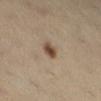| feature | finding |
|---|---|
| workup | catalogued during a skin exam; not biopsied |
| location | the left leg |
| subject | female, aged around 40 |
| image source | ~15 mm crop, total-body skin-cancer survey |